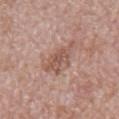| feature | finding |
|---|---|
| biopsy status | imaged on a skin check; not biopsied |
| site | the front of the torso |
| subject | male, approximately 65 years of age |
| imaging modality | 15 mm crop, total-body photography |
| lighting | white-light |
| lesion diameter | ≈5 mm |